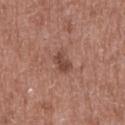The lesion was tiled from a total-body skin photograph and was not biopsied.
Imaged with white-light lighting.
A region of skin cropped from a whole-body photographic capture, roughly 15 mm wide.
The lesion is on the mid back.
A male subject, in their 50s.